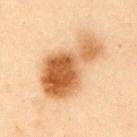Clinical summary:
The subject is a male in their mid- to late 50s. Measured at roughly 8.5 mm in maximum diameter. A 15 mm close-up extracted from a 3D total-body photography capture. The lesion is on the left upper arm. Imaged with cross-polarized lighting. An algorithmic analysis of the crop reported about 15 CIELAB-L* units darker than the surrounding skin and a normalized lesion–skin contrast near 10.5. It also reported a border-irregularity index near 5.5/10, a color-variation rating of about 8/10, and peripheral color asymmetry of about 3. The analysis additionally found a nevus-likeness score of about 100/100 and lesion-presence confidence of about 100/100.